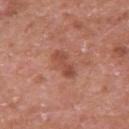{
  "patient": {
    "sex": "male",
    "age_approx": 65
  },
  "image": {
    "source": "total-body photography crop",
    "field_of_view_mm": 15
  },
  "automated_metrics": {
    "area_mm2_approx": 5.0,
    "eccentricity": 0.95,
    "shape_asymmetry": 0.3,
    "cielab_L": 49,
    "cielab_a": 26,
    "cielab_b": 30,
    "vs_skin_darker_L": 9.0,
    "vs_skin_contrast_norm": 6.5,
    "border_irregularity_0_10": 4.0,
    "color_variation_0_10": 2.0,
    "peripheral_color_asymmetry": 0.5,
    "nevus_likeness_0_100": 0,
    "lesion_detection_confidence_0_100": 100
  },
  "site": "left upper arm",
  "lesion_size": {
    "long_diameter_mm_approx": 4.0
  },
  "lighting": "white-light"
}